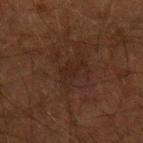No biopsy was performed on this lesion — it was imaged during a full skin examination and was not determined to be concerning.
An algorithmic analysis of the crop reported a mean CIELAB color near L≈17 a*≈14 b*≈18, roughly 3 lightness units darker than nearby skin, and a normalized lesion–skin contrast near 5.
Cropped from a total-body skin-imaging series; the visible field is about 15 mm.
The patient is a male in their 50s.
The lesion is located on the arm.